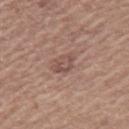Recorded during total-body skin imaging; not selected for excision or biopsy.
Located on the left upper arm.
The lesion's longest dimension is about 3 mm.
This is a white-light tile.
Automated tile analysis of the lesion measured a footprint of about 5 mm² and an outline eccentricity of about 0.65 (0 = round, 1 = elongated). The analysis additionally found border irregularity of about 2.5 on a 0–10 scale, a within-lesion color-variation index near 3.5/10, and a peripheral color-asymmetry measure near 1. The analysis additionally found a classifier nevus-likeness of about 0/100 and lesion-presence confidence of about 100/100.
Cropped from a whole-body photographic skin survey; the tile spans about 15 mm.
A male patient about 60 years old.